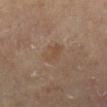Impression:
Recorded during total-body skin imaging; not selected for excision or biopsy.
Image and clinical context:
The subject is a male in their mid- to late 60s. The lesion is located on the right lower leg. This image is a 15 mm lesion crop taken from a total-body photograph.